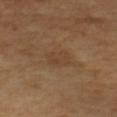Q: Lesion size?
A: ~3 mm (longest diameter)
Q: What are the patient's age and sex?
A: male, aged 63 to 67
Q: How was this image acquired?
A: total-body-photography crop, ~15 mm field of view
Q: What lighting was used for the tile?
A: cross-polarized illumination